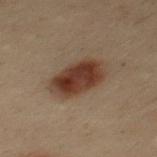Part of a total-body skin-imaging series; this lesion was reviewed on a skin check and was not flagged for biopsy. On the back. Longest diameter approximately 5.5 mm. Captured under cross-polarized illumination. The patient is a male aged 28–32. A 15 mm crop from a total-body photograph taken for skin-cancer surveillance.